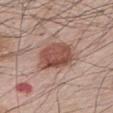Q: Was a biopsy performed?
A: catalogued during a skin exam; not biopsied
Q: What lighting was used for the tile?
A: white-light
Q: What is the lesion's diameter?
A: about 5.5 mm
Q: Automated lesion metrics?
A: a shape eccentricity near 0.75 and two-axis asymmetry of about 0.15; an average lesion color of about L≈50 a*≈23 b*≈26 (CIELAB), a lesion–skin lightness drop of about 13, and a normalized border contrast of about 9.5; border irregularity of about 2 on a 0–10 scale, internal color variation of about 4.5 on a 0–10 scale, and a peripheral color-asymmetry measure near 1.5; a nevus-likeness score of about 100/100 and lesion-presence confidence of about 100/100
Q: What are the patient's age and sex?
A: male, aged around 65
Q: How was this image acquired?
A: 15 mm crop, total-body photography
Q: Where on the body is the lesion?
A: the back